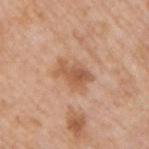Impression: This lesion was catalogued during total-body skin photography and was not selected for biopsy. Acquisition and patient details: A male subject, about 75 years old. The lesion is located on the right upper arm. This is a white-light tile. A 15 mm crop from a total-body photograph taken for skin-cancer surveillance.